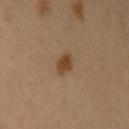The lesion was photographed on a routine skin check and not biopsied; there is no pathology result.
Captured under cross-polarized illumination.
The total-body-photography lesion software estimated two-axis asymmetry of about 0.3. The analysis additionally found an automated nevus-likeness rating near 100 out of 100 and lesion-presence confidence of about 100/100.
Located on the left upper arm.
Longest diameter approximately 2.5 mm.
A 15 mm close-up tile from a total-body photography series done for melanoma screening.
A male patient approximately 40 years of age.